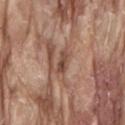Assessment:
The lesion was tiled from a total-body skin photograph and was not biopsied.
Acquisition and patient details:
From the lower back. An algorithmic analysis of the crop reported border irregularity of about 3 on a 0–10 scale, internal color variation of about 0 on a 0–10 scale, and radial color variation of about 0. And it measured a nevus-likeness score of about 10/100 and a detector confidence of about 90 out of 100 that the crop contains a lesion. A lesion tile, about 15 mm wide, cut from a 3D total-body photograph. Captured under white-light illumination. The patient is a male roughly 80 years of age.